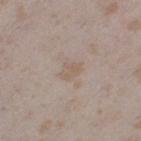The lesion was tiled from a total-body skin photograph and was not biopsied.
Located on the leg.
The lesion-visualizer software estimated an area of roughly 4.5 mm² and an eccentricity of roughly 0.65. And it measured roughly 6 lightness units darker than nearby skin and a normalized lesion–skin contrast near 5. The analysis additionally found a border-irregularity rating of about 2.5/10, a color-variation rating of about 1.5/10, and a peripheral color-asymmetry measure near 0.5. The analysis additionally found an automated nevus-likeness rating near 0 out of 100.
A close-up tile cropped from a whole-body skin photograph, about 15 mm across.
About 2.5 mm across.
The tile uses white-light illumination.
The patient is a female aged around 25.